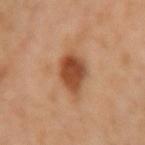The lesion was photographed on a routine skin check and not biopsied; there is no pathology result.
A 15 mm close-up tile from a total-body photography series done for melanoma screening.
A female subject, roughly 35 years of age.
Imaged with cross-polarized lighting.
An algorithmic analysis of the crop reported an area of roughly 10 mm², a shape eccentricity near 0.45, and a shape-asymmetry score of about 0.2 (0 = symmetric). The analysis additionally found a mean CIELAB color near L≈49 a*≈25 b*≈36 and roughly 14 lightness units darker than nearby skin. The software also gave a border-irregularity rating of about 1.5/10, a color-variation rating of about 4/10, and radial color variation of about 1. The software also gave an automated nevus-likeness rating near 100 out of 100 and lesion-presence confidence of about 100/100.
Measured at roughly 4 mm in maximum diameter.
Located on the arm.